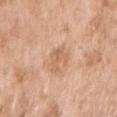| feature | finding |
|---|---|
| patient | female, in their mid- to late 70s |
| acquisition | total-body-photography crop, ~15 mm field of view |
| body site | the right upper arm |